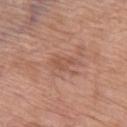{
  "biopsy_status": "not biopsied; imaged during a skin examination",
  "site": "left thigh",
  "lighting": "white-light",
  "lesion_size": {
    "long_diameter_mm_approx": 2.5
  },
  "automated_metrics": {
    "area_mm2_approx": 3.5,
    "eccentricity": 0.8,
    "shape_asymmetry": 0.35,
    "cielab_L": 53,
    "cielab_a": 23,
    "cielab_b": 29,
    "vs_skin_darker_L": 7.0,
    "peripheral_color_asymmetry": 0.5,
    "nevus_likeness_0_100": 0
  },
  "image": {
    "source": "total-body photography crop",
    "field_of_view_mm": 15
  },
  "patient": {
    "sex": "female",
    "age_approx": 70
  }
}